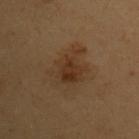Captured during whole-body skin photography for melanoma surveillance; the lesion was not biopsied. The recorded lesion diameter is about 5 mm. From the upper back. A female subject, aged around 60. Imaged with cross-polarized lighting. Automated tile analysis of the lesion measured a border-irregularity rating of about 5/10. It also reported a classifier nevus-likeness of about 20/100. Cropped from a whole-body photographic skin survey; the tile spans about 15 mm.